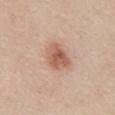Assessment:
Part of a total-body skin-imaging series; this lesion was reviewed on a skin check and was not flagged for biopsy.
Context:
The lesion is on the chest. A female patient, roughly 45 years of age. Cropped from a whole-body photographic skin survey; the tile spans about 15 mm. Imaged with white-light lighting.